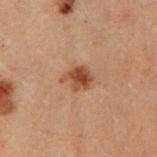Q: Was this lesion biopsied?
A: no biopsy performed (imaged during a skin exam)
Q: How was the tile lit?
A: cross-polarized
Q: What is the lesion's diameter?
A: ≈3 mm
Q: Who is the patient?
A: female, in their 30s
Q: How was this image acquired?
A: ~15 mm crop, total-body skin-cancer survey
Q: Lesion location?
A: the left arm